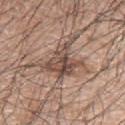biopsy status: imaged on a skin check; not biopsied
diameter: ≈4.5 mm
location: the left upper arm
patient: male, aged 68–72
image: ~15 mm crop, total-body skin-cancer survey
illumination: white-light illumination